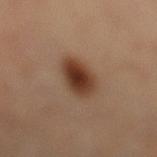Assessment: The lesion was photographed on a routine skin check and not biopsied; there is no pathology result. Clinical summary: An algorithmic analysis of the crop reported a lesion color around L≈38 a*≈20 b*≈29 in CIELAB, about 14 CIELAB-L* units darker than the surrounding skin, and a normalized border contrast of about 11.5. The software also gave a border-irregularity index near 2/10, a within-lesion color-variation index near 5.5/10, and radial color variation of about 1.5. On the left lower leg. This image is a 15 mm lesion crop taken from a total-body photograph. A female subject, in their mid-60s. The recorded lesion diameter is about 4.5 mm.